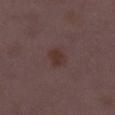Assessment: Part of a total-body skin-imaging series; this lesion was reviewed on a skin check and was not flagged for biopsy. Acquisition and patient details: On the left lower leg. A region of skin cropped from a whole-body photographic capture, roughly 15 mm wide. A female patient, approximately 30 years of age.